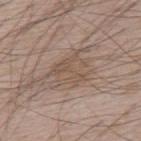Clinical impression:
No biopsy was performed on this lesion — it was imaged during a full skin examination and was not determined to be concerning.
Context:
Longest diameter approximately 6 mm. Captured under white-light illumination. Automated image analysis of the tile measured an outline eccentricity of about 0.75 (0 = round, 1 = elongated). And it measured a border-irregularity rating of about 8/10 and a within-lesion color-variation index near 3.5/10. The analysis additionally found a nevus-likeness score of about 0/100 and lesion-presence confidence of about 65/100. A male subject about 65 years old. Located on the upper back. A roughly 15 mm field-of-view crop from a total-body skin photograph.